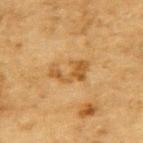The lesion was photographed on a routine skin check and not biopsied; there is no pathology result. From the upper back. A 15 mm close-up extracted from a 3D total-body photography capture. A male patient approximately 85 years of age or older. Imaged with cross-polarized lighting. The lesion-visualizer software estimated a lesion color around L≈48 a*≈18 b*≈40 in CIELAB and about 9 CIELAB-L* units darker than the surrounding skin. The software also gave a nevus-likeness score of about 40/100 and a lesion-detection confidence of about 100/100.